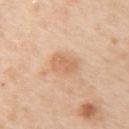<tbp_lesion>
<biopsy_status>not biopsied; imaged during a skin examination</biopsy_status>
<lighting>white-light</lighting>
<patient>
  <sex>female</sex>
  <age_approx>50</age_approx>
</patient>
<site>right upper arm</site>
<image>
  <source>total-body photography crop</source>
  <field_of_view_mm>15</field_of_view_mm>
</image>
</tbp_lesion>